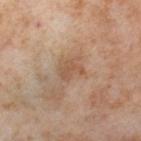Context: Longest diameter approximately 3 mm. The lesion is located on the right thigh. The total-body-photography lesion software estimated roughly 7 lightness units darker than nearby skin and a normalized border contrast of about 5.5. This image is a 15 mm lesion crop taken from a total-body photograph. Imaged with cross-polarized lighting. The patient is a female in their mid- to late 50s.